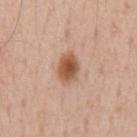Findings:
• workup · total-body-photography surveillance lesion; no biopsy
• tile lighting · white-light illumination
• diameter · about 4 mm
• image source · ~15 mm crop, total-body skin-cancer survey
• subject · male, aged 53–57
• anatomic site · the front of the torso
• automated lesion analysis · a mean CIELAB color near L≈55 a*≈22 b*≈33, about 14 CIELAB-L* units darker than the surrounding skin, and a normalized lesion–skin contrast near 10; a border-irregularity index near 1.5/10 and peripheral color asymmetry of about 1